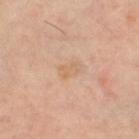Case summary:
- follow-up: catalogued during a skin exam; not biopsied
- TBP lesion metrics: an area of roughly 4 mm² and a shape eccentricity near 0.75; a border-irregularity index near 2/10, internal color variation of about 2.5 on a 0–10 scale, and peripheral color asymmetry of about 1; a nevus-likeness score of about 0/100 and a detector confidence of about 100 out of 100 that the crop contains a lesion
- tile lighting: cross-polarized illumination
- subject: female, aged 58–62
- site: the right thigh
- image source: ~15 mm tile from a whole-body skin photo